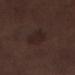Part of a total-body skin-imaging series; this lesion was reviewed on a skin check and was not flagged for biopsy. Located on the left lower leg. A roughly 15 mm field-of-view crop from a total-body skin photograph. This is a white-light tile. Measured at roughly 3.5 mm in maximum diameter. A male subject, aged approximately 70.